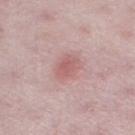image: ~15 mm crop, total-body skin-cancer survey | subject: female, in their mid- to late 40s | site: the leg.